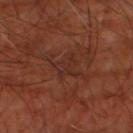Clinical impression: Part of a total-body skin-imaging series; this lesion was reviewed on a skin check and was not flagged for biopsy. Image and clinical context: The total-body-photography lesion software estimated a lesion area of about 5.5 mm². The software also gave a border-irregularity rating of about 6.5/10 and a color-variation rating of about 2/10. Located on the left thigh. A subject aged approximately 65. A lesion tile, about 15 mm wide, cut from a 3D total-body photograph. Captured under cross-polarized illumination. Longest diameter approximately 3 mm.